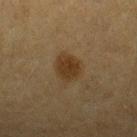Part of a total-body skin-imaging series; this lesion was reviewed on a skin check and was not flagged for biopsy. The subject is a female aged around 55. Longest diameter approximately 3.5 mm. A 15 mm close-up tile from a total-body photography series done for melanoma screening. Automated image analysis of the tile measured a lesion area of about 6.5 mm² and a symmetry-axis asymmetry near 0.3. The analysis additionally found a border-irregularity rating of about 2.5/10, internal color variation of about 2 on a 0–10 scale, and peripheral color asymmetry of about 0.5. On the left forearm.